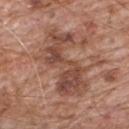This lesion was catalogued during total-body skin photography and was not selected for biopsy. Cropped from a whole-body photographic skin survey; the tile spans about 15 mm. On the upper back. A male patient, about 80 years old. Captured under white-light illumination. The lesion's longest dimension is about 8.5 mm. Automated tile analysis of the lesion measured a lesion area of about 25 mm². The analysis additionally found a lesion color around L≈47 a*≈22 b*≈28 in CIELAB, a lesion–skin lightness drop of about 10, and a normalized border contrast of about 7.5.